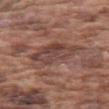Captured during whole-body skin photography for melanoma surveillance; the lesion was not biopsied.
A male patient, about 75 years old.
The total-body-photography lesion software estimated a footprint of about 19 mm², a shape eccentricity near 0.75, and two-axis asymmetry of about 0.3. The software also gave a mean CIELAB color near L≈44 a*≈20 b*≈24, about 8 CIELAB-L* units darker than the surrounding skin, and a normalized lesion–skin contrast near 6.5. The analysis additionally found a border-irregularity rating of about 3.5/10, a color-variation rating of about 6/10, and radial color variation of about 2. The software also gave a lesion-detection confidence of about 70/100.
Located on the arm.
A close-up tile cropped from a whole-body skin photograph, about 15 mm across.
The tile uses white-light illumination.
Longest diameter approximately 6 mm.